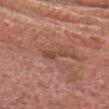Background: Measured at roughly 3.5 mm in maximum diameter. A male patient, about 65 years old. The lesion is on the head or neck. The total-body-photography lesion software estimated an area of roughly 4 mm², an outline eccentricity of about 0.95 (0 = round, 1 = elongated), and two-axis asymmetry of about 0.4. A 15 mm crop from a total-body photograph taken for skin-cancer surveillance. This is a white-light tile.